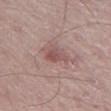Captured during whole-body skin photography for melanoma surveillance; the lesion was not biopsied.
Imaged with white-light lighting.
From the left thigh.
A male patient, aged approximately 50.
Longest diameter approximately 3 mm.
A region of skin cropped from a whole-body photographic capture, roughly 15 mm wide.
An algorithmic analysis of the crop reported a lesion area of about 4 mm² and a symmetry-axis asymmetry near 0.45. It also reported about 9 CIELAB-L* units darker than the surrounding skin and a normalized border contrast of about 6.5. The software also gave a border-irregularity index near 4.5/10, a color-variation rating of about 2/10, and peripheral color asymmetry of about 0.5. And it measured a nevus-likeness score of about 15/100 and a lesion-detection confidence of about 100/100.